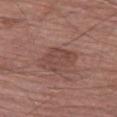{"patient": {"sex": "male", "age_approx": 75}, "site": "left thigh", "image": {"source": "total-body photography crop", "field_of_view_mm": 15}, "lighting": "white-light", "lesion_size": {"long_diameter_mm_approx": 4.0}}